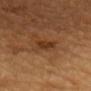No biopsy was performed on this lesion — it was imaged during a full skin examination and was not determined to be concerning.
Longest diameter approximately 2.5 mm.
The lesion is located on the chest.
This image is a 15 mm lesion crop taken from a total-body photograph.
The lesion-visualizer software estimated an area of roughly 3.5 mm², an eccentricity of roughly 0.75, and a shape-asymmetry score of about 0.3 (0 = symmetric). It also reported about 8 CIELAB-L* units darker than the surrounding skin. And it measured border irregularity of about 2.5 on a 0–10 scale, internal color variation of about 1.5 on a 0–10 scale, and radial color variation of about 0.5. The analysis additionally found an automated nevus-likeness rating near 25 out of 100 and a detector confidence of about 100 out of 100 that the crop contains a lesion.
The subject is a male in their mid- to late 80s or older.